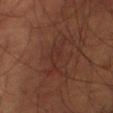notes = no biopsy performed (imaged during a skin exam) | automated lesion analysis = a lesion area of about 6.5 mm²; a nevus-likeness score of about 5/100 and a lesion-detection confidence of about 95/100 | illumination = cross-polarized illumination | lesion size = ≈5.5 mm | patient = male, aged 58–62 | imaging modality = total-body-photography crop, ~15 mm field of view.